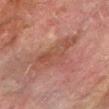| field | value |
|---|---|
| notes | catalogued during a skin exam; not biopsied |
| automated lesion analysis | a border-irregularity rating of about 4.5/10, internal color variation of about 3 on a 0–10 scale, and a peripheral color-asymmetry measure near 1; a detector confidence of about 100 out of 100 that the crop contains a lesion |
| lesion size | ≈6.5 mm |
| imaging modality | total-body-photography crop, ~15 mm field of view |
| body site | the right forearm |
| patient | male, aged approximately 75 |